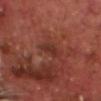The lesion was photographed on a routine skin check and not biopsied; there is no pathology result.
The lesion is located on the head or neck.
The patient is a male roughly 70 years of age.
Captured under cross-polarized illumination.
Approximately 3 mm at its widest.
A roughly 15 mm field-of-view crop from a total-body skin photograph.